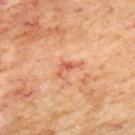<tbp_lesion>
  <biopsy_status>not biopsied; imaged during a skin examination</biopsy_status>
  <site>arm</site>
  <automated_metrics>
    <color_variation_0_10>0.0</color_variation_0_10>
    <peripheral_color_asymmetry>0.0</peripheral_color_asymmetry>
  </automated_metrics>
  <lesion_size>
    <long_diameter_mm_approx>3.0</long_diameter_mm_approx>
  </lesion_size>
  <lighting>cross-polarized</lighting>
  <image>
    <source>total-body photography crop</source>
    <field_of_view_mm>15</field_of_view_mm>
  </image>
  <patient>
    <sex>female</sex>
    <age_approx>50</age_approx>
  </patient>
</tbp_lesion>